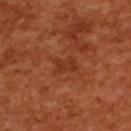Q: Is there a histopathology result?
A: total-body-photography surveillance lesion; no biopsy
Q: What is the lesion's diameter?
A: about 2.5 mm
Q: What is the anatomic site?
A: the upper back
Q: What did automated image analysis measure?
A: a mean CIELAB color near L≈37 a*≈29 b*≈37 and a normalized lesion–skin contrast near 5.5; a border-irregularity index near 3.5/10, a within-lesion color-variation index near 1/10, and a peripheral color-asymmetry measure near 0.5
Q: How was the tile lit?
A: cross-polarized
Q: What are the patient's age and sex?
A: female, approximately 55 years of age
Q: How was this image acquired?
A: ~15 mm tile from a whole-body skin photo